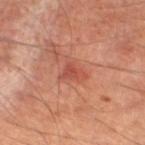This lesion was catalogued during total-body skin photography and was not selected for biopsy. Located on the left lower leg. A close-up tile cropped from a whole-body skin photograph, about 15 mm across. The tile uses cross-polarized illumination. An algorithmic analysis of the crop reported a lesion–skin lightness drop of about 8. The software also gave a border-irregularity index near 4.5/10, a color-variation rating of about 1.5/10, and radial color variation of about 0.5. The software also gave a classifier nevus-likeness of about 25/100 and lesion-presence confidence of about 100/100. A male patient, aged around 65.